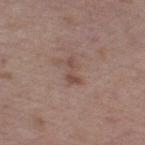Clinical impression:
Captured during whole-body skin photography for melanoma surveillance; the lesion was not biopsied.
Acquisition and patient details:
Longest diameter approximately 3 mm. Located on the leg. The subject is a female roughly 50 years of age. The tile uses white-light illumination. A 15 mm close-up extracted from a 3D total-body photography capture.